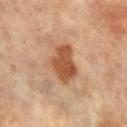This lesion was catalogued during total-body skin photography and was not selected for biopsy. The lesion is on the left leg. The subject is a female approximately 65 years of age. A 15 mm close-up tile from a total-body photography series done for melanoma screening.